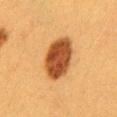Case summary:
– follow-up — total-body-photography surveillance lesion; no biopsy
– anatomic site — the mid back
– size — about 6 mm
– acquisition — 15 mm crop, total-body photography
– patient — female, about 40 years old
– tile lighting — cross-polarized illumination